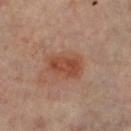Impression: Recorded during total-body skin imaging; not selected for excision or biopsy. Context: A female subject aged 58–62. The lesion-visualizer software estimated a lesion area of about 11 mm², a shape eccentricity near 0.75, and a symmetry-axis asymmetry near 0.2. It also reported a lesion color around L≈47 a*≈23 b*≈31 in CIELAB, a lesion–skin lightness drop of about 9, and a normalized lesion–skin contrast near 7.5. And it measured border irregularity of about 2 on a 0–10 scale and radial color variation of about 1. And it measured a classifier nevus-likeness of about 75/100. The lesion's longest dimension is about 4.5 mm. The lesion is located on the leg. A roughly 15 mm field-of-view crop from a total-body skin photograph.